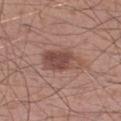Automated image analysis of the tile measured about 10 CIELAB-L* units darker than the surrounding skin and a normalized lesion–skin contrast near 7.5. Cropped from a total-body skin-imaging series; the visible field is about 15 mm. Measured at roughly 4.5 mm in maximum diameter. Captured under white-light illumination. A male patient, in their mid-50s. Located on the right lower leg.